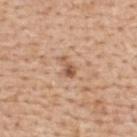Q: Is there a histopathology result?
A: total-body-photography surveillance lesion; no biopsy
Q: How was this image acquired?
A: total-body-photography crop, ~15 mm field of view
Q: How was the tile lit?
A: white-light illumination
Q: Automated lesion metrics?
A: a mean CIELAB color near L≈55 a*≈22 b*≈32, roughly 12 lightness units darker than nearby skin, and a normalized border contrast of about 8; a within-lesion color-variation index near 2/10
Q: What are the patient's age and sex?
A: female, aged 48–52
Q: Where on the body is the lesion?
A: the upper back
Q: What is the lesion's diameter?
A: ~2.5 mm (longest diameter)